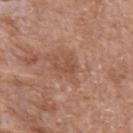Captured during whole-body skin photography for melanoma surveillance; the lesion was not biopsied.
Automated image analysis of the tile measured an average lesion color of about L≈49 a*≈22 b*≈30 (CIELAB) and about 7 CIELAB-L* units darker than the surrounding skin. The analysis additionally found a border-irregularity rating of about 6.5/10, a within-lesion color-variation index near 0/10, and peripheral color asymmetry of about 0. The analysis additionally found a detector confidence of about 100 out of 100 that the crop contains a lesion.
A 15 mm close-up extracted from a 3D total-body photography capture.
About 2.5 mm across.
Captured under white-light illumination.
Located on the abdomen.
A male patient aged 63 to 67.